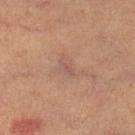follow-up=catalogued during a skin exam; not biopsied | location=the left lower leg | imaging modality=~15 mm crop, total-body skin-cancer survey | subject=female, aged around 60.